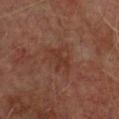Located on the chest.
This is a cross-polarized tile.
Automated tile analysis of the lesion measured a footprint of about 4.5 mm², an outline eccentricity of about 0.8 (0 = round, 1 = elongated), and two-axis asymmetry of about 0.45. The analysis additionally found a mean CIELAB color near L≈27 a*≈19 b*≈23, a lesion–skin lightness drop of about 5, and a normalized border contrast of about 5.5. The analysis additionally found a border-irregularity index near 5.5/10 and radial color variation of about 0.
A 15 mm close-up extracted from a 3D total-body photography capture.
The patient is a male aged 73–77.